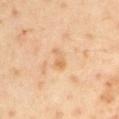<record>
<biopsy_status>not biopsied; imaged during a skin examination</biopsy_status>
<automated_metrics>
  <eccentricity>0.9</eccentricity>
  <border_irregularity_0_10>4.5</border_irregularity_0_10>
  <color_variation_0_10>0.5</color_variation_0_10>
  <peripheral_color_asymmetry>0.0</peripheral_color_asymmetry>
</automated_metrics>
<image>
  <source>total-body photography crop</source>
  <field_of_view_mm>15</field_of_view_mm>
</image>
<site>left upper arm</site>
<lighting>cross-polarized</lighting>
<lesion_size>
  <long_diameter_mm_approx>2.5</long_diameter_mm_approx>
</lesion_size>
<patient>
  <sex>male</sex>
  <age_approx>45</age_approx>
</patient>
</record>